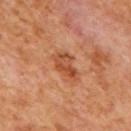{"biopsy_status": "not biopsied; imaged during a skin examination", "lesion_size": {"long_diameter_mm_approx": 4.0}, "patient": {"sex": "male", "age_approx": 65}, "image": {"source": "total-body photography crop", "field_of_view_mm": 15}, "site": "mid back"}